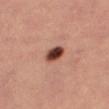<tbp_lesion>
  <biopsy_status>not biopsied; imaged during a skin examination</biopsy_status>
  <image>
    <source>total-body photography crop</source>
    <field_of_view_mm>15</field_of_view_mm>
  </image>
  <lesion_size>
    <long_diameter_mm_approx>3.0</long_diameter_mm_approx>
  </lesion_size>
  <lighting>cross-polarized</lighting>
  <automated_metrics>
    <border_irregularity_0_10>1.5</border_irregularity_0_10>
    <color_variation_0_10>5.5</color_variation_0_10>
    <peripheral_color_asymmetry>1.5</peripheral_color_asymmetry>
  </automated_metrics>
  <patient>
    <sex>female</sex>
    <age_approx>45</age_approx>
  </patient>
  <site>right thigh</site>
</tbp_lesion>